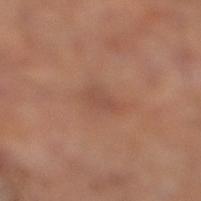Findings:
* biopsy status · total-body-photography surveillance lesion; no biopsy
* patient · male, aged around 65
* lesion diameter · ≈3 mm
* site · the right lower leg
* image source · total-body-photography crop, ~15 mm field of view
* tile lighting · cross-polarized illumination